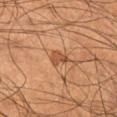Recorded during total-body skin imaging; not selected for excision or biopsy.